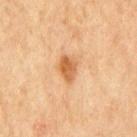notes = total-body-photography surveillance lesion; no biopsy | lighting = cross-polarized illumination | anatomic site = the mid back | patient = male, about 65 years old | size = ~3 mm (longest diameter) | imaging modality = 15 mm crop, total-body photography.